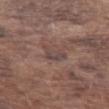This lesion was catalogued during total-body skin photography and was not selected for biopsy. This is a white-light tile. An algorithmic analysis of the crop reported a border-irregularity index near 4.5/10, a within-lesion color-variation index near 2/10, and a peripheral color-asymmetry measure near 0.5. A close-up tile cropped from a whole-body skin photograph, about 15 mm across. A male subject approximately 75 years of age. From the left forearm.